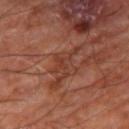Imaged during a routine full-body skin examination; the lesion was not biopsied and no histopathology is available. Located on the right thigh. The tile uses cross-polarized illumination. A 15 mm crop from a total-body photograph taken for skin-cancer surveillance. The subject is in their mid-60s. The lesion's longest dimension is about 3 mm.